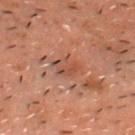Context:
Captured under cross-polarized illumination. A male subject about 50 years old. Cropped from a whole-body photographic skin survey; the tile spans about 15 mm. The lesion is on the chest. The lesion's longest dimension is about 3.5 mm. The lesion-visualizer software estimated a border-irregularity index near 2/10, a within-lesion color-variation index near 3.5/10, and radial color variation of about 1.